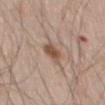Assessment:
This lesion was catalogued during total-body skin photography and was not selected for biopsy.
Background:
The total-body-photography lesion software estimated a footprint of about 4.5 mm². The software also gave border irregularity of about 1.5 on a 0–10 scale and a peripheral color-asymmetry measure near 1. A region of skin cropped from a whole-body photographic capture, roughly 15 mm wide. A male subject aged approximately 60. The lesion is located on the left thigh. Approximately 3 mm at its widest.